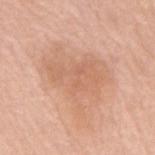Q: Was a biopsy performed?
A: imaged on a skin check; not biopsied
Q: What are the patient's age and sex?
A: female, aged 73 to 77
Q: What is the imaging modality?
A: ~15 mm tile from a whole-body skin photo
Q: Where on the body is the lesion?
A: the mid back
Q: Lesion size?
A: ≈7.5 mm
Q: How was the tile lit?
A: white-light